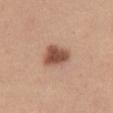Impression:
No biopsy was performed on this lesion — it was imaged during a full skin examination and was not determined to be concerning.
Background:
A female subject, aged around 25. A 15 mm crop from a total-body photograph taken for skin-cancer surveillance. The lesion is on the left thigh.